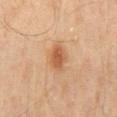The lesion was tiled from a total-body skin photograph and was not biopsied. An algorithmic analysis of the crop reported a shape eccentricity near 0.7 and a symmetry-axis asymmetry near 0.2. It also reported a lesion–skin lightness drop of about 10 and a normalized lesion–skin contrast near 7.5. The software also gave a border-irregularity index near 1.5/10, a color-variation rating of about 3/10, and radial color variation of about 1. A close-up tile cropped from a whole-body skin photograph, about 15 mm across. Measured at roughly 3 mm in maximum diameter. On the mid back. Captured under cross-polarized illumination. A male patient roughly 45 years of age.